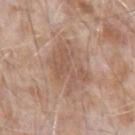Impression:
Part of a total-body skin-imaging series; this lesion was reviewed on a skin check and was not flagged for biopsy.
Image and clinical context:
From the arm. The patient is a male approximately 75 years of age. This image is a 15 mm lesion crop taken from a total-body photograph. Captured under white-light illumination. Approximately 5.5 mm at its widest. An algorithmic analysis of the crop reported an area of roughly 15 mm², an eccentricity of roughly 0.75, and two-axis asymmetry of about 0.3. It also reported a lesion color around L≈55 a*≈18 b*≈28 in CIELAB, a lesion–skin lightness drop of about 8, and a lesion-to-skin contrast of about 5.5 (normalized; higher = more distinct). It also reported a border-irregularity rating of about 5.5/10, internal color variation of about 3 on a 0–10 scale, and radial color variation of about 1.